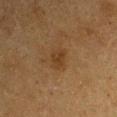Clinical impression: This lesion was catalogued during total-body skin photography and was not selected for biopsy. Image and clinical context: An algorithmic analysis of the crop reported a lesion color around L≈30 a*≈15 b*≈29 in CIELAB and a lesion–skin lightness drop of about 6. The analysis additionally found border irregularity of about 2.5 on a 0–10 scale, a color-variation rating of about 2/10, and peripheral color asymmetry of about 0.5. The software also gave a nevus-likeness score of about 40/100. The recorded lesion diameter is about 2.5 mm. A male patient, aged 73–77. The lesion is on the left upper arm. A 15 mm close-up extracted from a 3D total-body photography capture. Captured under cross-polarized illumination.